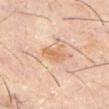Impression:
No biopsy was performed on this lesion — it was imaged during a full skin examination and was not determined to be concerning.
Acquisition and patient details:
A roughly 15 mm field-of-view crop from a total-body skin photograph. Located on the front of the torso. Measured at roughly 3 mm in maximum diameter. A male subject aged 58–62.